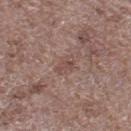The lesion was photographed on a routine skin check and not biopsied; there is no pathology result.
Automated image analysis of the tile measured a footprint of about 3.5 mm² and an outline eccentricity of about 0.8 (0 = round, 1 = elongated). And it measured a mean CIELAB color near L≈48 a*≈18 b*≈22 and a lesion-to-skin contrast of about 5 (normalized; higher = more distinct). It also reported a border-irregularity rating of about 2.5/10, a within-lesion color-variation index near 2/10, and peripheral color asymmetry of about 0.5.
The tile uses white-light illumination.
The recorded lesion diameter is about 2.5 mm.
A roughly 15 mm field-of-view crop from a total-body skin photograph.
A male patient, approximately 70 years of age.
Located on the left lower leg.